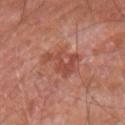<tbp_lesion>
  <biopsy_status>not biopsied; imaged during a skin examination</biopsy_status>
  <site>right upper arm</site>
  <image>
    <source>total-body photography crop</source>
    <field_of_view_mm>15</field_of_view_mm>
  </image>
  <patient>
    <sex>male</sex>
    <age_approx>60</age_approx>
  </patient>
</tbp_lesion>